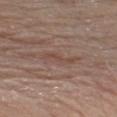follow-up: imaged on a skin check; not biopsied | acquisition: ~15 mm crop, total-body skin-cancer survey | site: the chest | subject: male, approximately 75 years of age | diameter: about 3.5 mm | illumination: white-light.